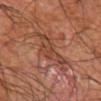No biopsy was performed on this lesion — it was imaged during a full skin examination and was not determined to be concerning. Captured under cross-polarized illumination. Located on the left upper arm. The patient is a male roughly 65 years of age. A 15 mm close-up tile from a total-body photography series done for melanoma screening. Measured at roughly 6.5 mm in maximum diameter.